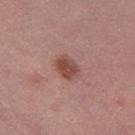biopsy status — catalogued during a skin exam; not biopsied
body site — the left thigh
lighting — white-light
diameter — ≈3 mm
patient — female, roughly 55 years of age
automated lesion analysis — a footprint of about 6.5 mm² and a shape-asymmetry score of about 0.2 (0 = symmetric); border irregularity of about 1.5 on a 0–10 scale, internal color variation of about 2.5 on a 0–10 scale, and a peripheral color-asymmetry measure near 1
image — 15 mm crop, total-body photography